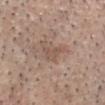Findings:
* acquisition — 15 mm crop, total-body photography
* body site — the head or neck
* TBP lesion metrics — a lesion area of about 4 mm², an outline eccentricity of about 0.75 (0 = round, 1 = elongated), and two-axis asymmetry of about 0.5; internal color variation of about 1.5 on a 0–10 scale; a nevus-likeness score of about 0/100 and lesion-presence confidence of about 95/100
* size — ~3 mm (longest diameter)
* subject — male, aged around 80